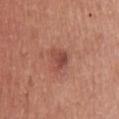Findings:
* biopsy status · catalogued during a skin exam; not biopsied
* location · the upper back
* image source · ~15 mm tile from a whole-body skin photo
* tile lighting · white-light
* patient · male, in their 60s
* size · ≈2.5 mm